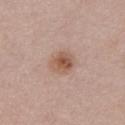Assessment: Imaged during a routine full-body skin examination; the lesion was not biopsied and no histopathology is available. Context: The subject is a female aged 48–52. The lesion is on the chest. This is a white-light tile. A lesion tile, about 15 mm wide, cut from a 3D total-body photograph.